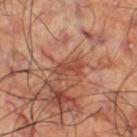<record>
  <biopsy_status>not biopsied; imaged during a skin examination</biopsy_status>
  <lighting>cross-polarized</lighting>
  <lesion_size>
    <long_diameter_mm_approx>3.5</long_diameter_mm_approx>
  </lesion_size>
  <site>leg</site>
  <image>
    <source>total-body photography crop</source>
    <field_of_view_mm>15</field_of_view_mm>
  </image>
  <patient>
    <sex>male</sex>
    <age_approx>60</age_approx>
  </patient>
  <automated_metrics>
    <area_mm2_approx>6.0</area_mm2_approx>
    <eccentricity>0.8</eccentricity>
    <shape_asymmetry>0.35</shape_asymmetry>
    <nevus_likeness_0_100>0</nevus_likeness_0_100>
    <lesion_detection_confidence_0_100>75</lesion_detection_confidence_0_100>
  </automated_metrics>
</record>